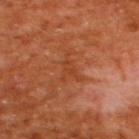The lesion was photographed on a routine skin check and not biopsied; there is no pathology result.
Located on the upper back.
Measured at roughly 3.5 mm in maximum diameter.
Automated tile analysis of the lesion measured border irregularity of about 7 on a 0–10 scale, a within-lesion color-variation index near 1/10, and a peripheral color-asymmetry measure near 0.5.
This is a cross-polarized tile.
A male patient, aged 63–67.
A roughly 15 mm field-of-view crop from a total-body skin photograph.